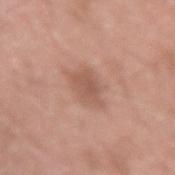Notes:
- follow-up — no biopsy performed (imaged during a skin exam)
- patient — male, aged approximately 20
- image — ~15 mm crop, total-body skin-cancer survey
- site — the right upper arm